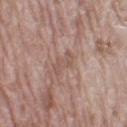  biopsy_status: not biopsied; imaged during a skin examination
  automated_metrics:
    area_mm2_approx: 3.5
    eccentricity: 0.95
    shape_asymmetry: 0.55
    cielab_L: 53
    cielab_a: 18
    cielab_b: 24
    vs_skin_darker_L: 7.0
    vs_skin_contrast_norm: 5.0
    nevus_likeness_0_100: 0
  image:
    source: total-body photography crop
    field_of_view_mm: 15
  lesion_size:
    long_diameter_mm_approx: 3.5
  site: left thigh
  patient:
    sex: female
    age_approx: 75
  lighting: white-light